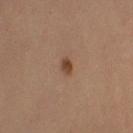workup: total-body-photography surveillance lesion; no biopsy
size: ~2 mm (longest diameter)
location: the right forearm
image: ~15 mm crop, total-body skin-cancer survey
patient: female, about 35 years old
TBP lesion metrics: a lesion color around L≈41 a*≈19 b*≈29 in CIELAB and a lesion–skin lightness drop of about 10; border irregularity of about 2.5 on a 0–10 scale and a color-variation rating of about 2/10
tile lighting: cross-polarized